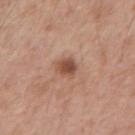This lesion was catalogued during total-body skin photography and was not selected for biopsy. The lesion's longest dimension is about 3 mm. Imaged with white-light lighting. Located on the arm. The total-body-photography lesion software estimated an average lesion color of about L≈51 a*≈22 b*≈29 (CIELAB) and a normalized lesion–skin contrast near 8. The analysis additionally found a border-irregularity index near 2.5/10. A male patient, aged 53 to 57. A close-up tile cropped from a whole-body skin photograph, about 15 mm across.